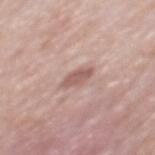The lesion was tiled from a total-body skin photograph and was not biopsied.
A male patient, aged approximately 55.
Located on the mid back.
A lesion tile, about 15 mm wide, cut from a 3D total-body photograph.